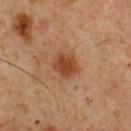follow-up = total-body-photography surveillance lesion; no biopsy
patient = male, approximately 75 years of age
imaging modality = total-body-photography crop, ~15 mm field of view
location = the chest